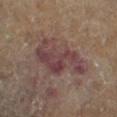<tbp_lesion>
<biopsy_status>not biopsied; imaged during a skin examination</biopsy_status>
<automated_metrics>
  <nevus_likeness_0_100>0</nevus_likeness_0_100>
  <lesion_detection_confidence_0_100>100</lesion_detection_confidence_0_100>
</automated_metrics>
<site>right lower leg</site>
<lesion_size>
  <long_diameter_mm_approx>7.0</long_diameter_mm_approx>
</lesion_size>
<image>
  <source>total-body photography crop</source>
  <field_of_view_mm>15</field_of_view_mm>
</image>
<lighting>cross-polarized</lighting>
<patient>
  <sex>male</sex>
  <age_approx>85+</age_approx>
</patient>
</tbp_lesion>